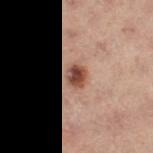Clinical impression:
Recorded during total-body skin imaging; not selected for excision or biopsy.
Background:
A roughly 15 mm field-of-view crop from a total-body skin photograph. From the left thigh. The subject is a female in their mid-50s. The tile uses cross-polarized illumination.